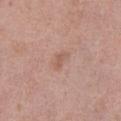tile lighting: white-light
site: the right thigh
subject: female, approximately 40 years of age
diameter: ~2.5 mm (longest diameter)
TBP lesion metrics: a mean CIELAB color near L≈57 a*≈21 b*≈28, about 7 CIELAB-L* units darker than the surrounding skin, and a normalized lesion–skin contrast near 5.5; a border-irregularity index near 4.5/10, a within-lesion color-variation index near 0/10, and peripheral color asymmetry of about 0
acquisition: ~15 mm crop, total-body skin-cancer survey